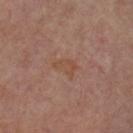follow-up: total-body-photography surveillance lesion; no biopsy | lesion diameter: ~3 mm (longest diameter) | lighting: cross-polarized illumination | location: the left upper arm | subject: male, aged 63–67 | image: ~15 mm crop, total-body skin-cancer survey.